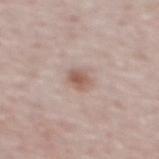<tbp_lesion>
  <biopsy_status>not biopsied; imaged during a skin examination</biopsy_status>
  <lesion_size>
    <long_diameter_mm_approx>2.5</long_diameter_mm_approx>
  </lesion_size>
  <patient>
    <sex>male</sex>
    <age_approx>50</age_approx>
  </patient>
  <image>
    <source>total-body photography crop</source>
    <field_of_view_mm>15</field_of_view_mm>
  </image>
  <site>upper back</site>
  <lighting>white-light</lighting>
</tbp_lesion>